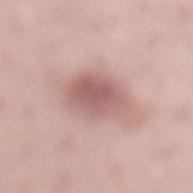Clinical impression: Part of a total-body skin-imaging series; this lesion was reviewed on a skin check and was not flagged for biopsy.